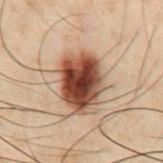follow-up: no biopsy performed (imaged during a skin exam); anatomic site: the abdomen; lesion size: ≈6 mm; subject: male, approximately 50 years of age; image source: 15 mm crop, total-body photography; tile lighting: cross-polarized.